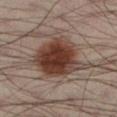Recorded during total-body skin imaging; not selected for excision or biopsy.
The lesion is located on the left lower leg.
The patient is a male in their 40s.
A 15 mm close-up tile from a total-body photography series done for melanoma screening.
Captured under cross-polarized illumination.
The lesion's longest dimension is about 7.5 mm.